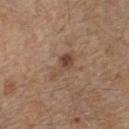Q: Is there a histopathology result?
A: no biopsy performed (imaged during a skin exam)